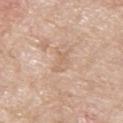Part of a total-body skin-imaging series; this lesion was reviewed on a skin check and was not flagged for biopsy. A male subject, aged around 65. A 15 mm close-up extracted from a 3D total-body photography capture. Imaged with white-light lighting. Automated tile analysis of the lesion measured a mean CIELAB color near L≈64 a*≈17 b*≈31 and a normalized border contrast of about 5. The software also gave border irregularity of about 6.5 on a 0–10 scale, internal color variation of about 0 on a 0–10 scale, and peripheral color asymmetry of about 0. And it measured a detector confidence of about 95 out of 100 that the crop contains a lesion. Measured at roughly 3 mm in maximum diameter. Located on the upper back.